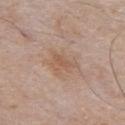Q: Is there a histopathology result?
A: imaged on a skin check; not biopsied
Q: What is the anatomic site?
A: the front of the torso
Q: Lesion size?
A: ≈3.5 mm
Q: How was this image acquired?
A: 15 mm crop, total-body photography
Q: What are the patient's age and sex?
A: male, aged approximately 55
Q: Illumination type?
A: white-light
Q: What did automated image analysis measure?
A: a lesion area of about 5.5 mm², an eccentricity of roughly 0.8, and a shape-asymmetry score of about 0.4 (0 = symmetric); an average lesion color of about L≈56 a*≈18 b*≈29 (CIELAB), a lesion–skin lightness drop of about 7, and a lesion-to-skin contrast of about 5.5 (normalized; higher = more distinct); internal color variation of about 1.5 on a 0–10 scale and peripheral color asymmetry of about 0.5